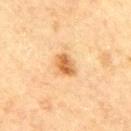biopsy_status: not biopsied; imaged during a skin examination
patient:
  sex: male
  age_approx: 45
lighting: cross-polarized
site: right upper arm
image:
  source: total-body photography crop
  field_of_view_mm: 15
lesion_size:
  long_diameter_mm_approx: 3.0